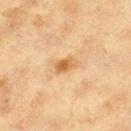Context:
A 15 mm close-up extracted from a 3D total-body photography capture. The subject is a female roughly 40 years of age. Approximately 3 mm at its widest. The lesion is on the left thigh. Automated tile analysis of the lesion measured a shape eccentricity near 0.75 and two-axis asymmetry of about 0.2. And it measured a lesion color around L≈55 a*≈19 b*≈38 in CIELAB, roughly 10 lightness units darker than nearby skin, and a normalized border contrast of about 7.5. The analysis additionally found a color-variation rating of about 4/10 and radial color variation of about 1.5. The software also gave a lesion-detection confidence of about 100/100. This is a cross-polarized tile.